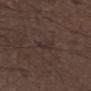follow-up = catalogued during a skin exam; not biopsied | illumination = white-light | image = total-body-photography crop, ~15 mm field of view | location = the right lower leg | subject = male, aged 48 to 52.